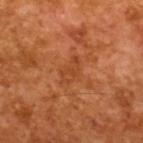biopsy_status: not biopsied; imaged during a skin examination
lighting: cross-polarized
lesion_size:
  long_diameter_mm_approx: 3.0
patient:
  sex: male
  age_approx: 65
automated_metrics:
  vs_skin_contrast_norm: 5.0
  nevus_likeness_0_100: 0
  lesion_detection_confidence_0_100: 100
image:
  source: total-body photography crop
  field_of_view_mm: 15